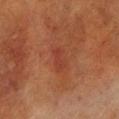{
  "biopsy_status": "not biopsied; imaged during a skin examination"
}